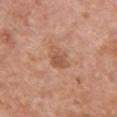The lesion was tiled from a total-body skin photograph and was not biopsied. A female subject, aged approximately 50. A 15 mm crop from a total-body photograph taken for skin-cancer surveillance. Measured at roughly 3 mm in maximum diameter. The tile uses white-light illumination. From the chest.